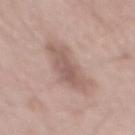{
  "biopsy_status": "not biopsied; imaged during a skin examination",
  "patient": {
    "sex": "male",
    "age_approx": 55
  },
  "lighting": "white-light",
  "site": "mid back",
  "lesion_size": {
    "long_diameter_mm_approx": 6.5
  },
  "image": {
    "source": "total-body photography crop",
    "field_of_view_mm": 15
  }
}